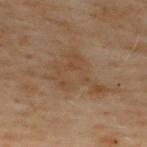On the upper back.
A male patient, aged around 50.
A 15 mm close-up tile from a total-body photography series done for melanoma screening.
The lesion-visualizer software estimated an outline eccentricity of about 0.65 (0 = round, 1 = elongated) and a shape-asymmetry score of about 0.55 (0 = symmetric). The software also gave an average lesion color of about L≈37 a*≈13 b*≈26 (CIELAB) and a lesion-to-skin contrast of about 5 (normalized; higher = more distinct). The analysis additionally found border irregularity of about 7 on a 0–10 scale, a within-lesion color-variation index near 2.5/10, and radial color variation of about 0.5. The software also gave a detector confidence of about 100 out of 100 that the crop contains a lesion.
The tile uses cross-polarized illumination.
The lesion's longest dimension is about 6 mm.